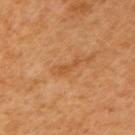Notes:
• follow-up — no biopsy performed (imaged during a skin exam)
• lighting — cross-polarized illumination
• patient — female, approximately 50 years of age
• acquisition — ~15 mm tile from a whole-body skin photo
• body site — the right upper arm